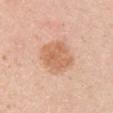• biopsy status · imaged on a skin check; not biopsied
• illumination · white-light
• location · the right upper arm
• image · ~15 mm crop, total-body skin-cancer survey
• patient · female, aged 28–32
• image-analysis metrics · a footprint of about 13 mm²; a classifier nevus-likeness of about 20/100
• size · ~4.5 mm (longest diameter)